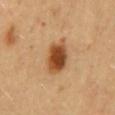| key | value |
|---|---|
| imaging modality | ~15 mm tile from a whole-body skin photo |
| location | the mid back |
| subject | female, in their 60s |
| image-analysis metrics | peripheral color asymmetry of about 1.5; a nevus-likeness score of about 100/100 |
| illumination | cross-polarized illumination |
| size | ≈4.5 mm |